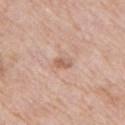A close-up tile cropped from a whole-body skin photograph, about 15 mm across. A female subject in their 70s. Located on the right upper arm.